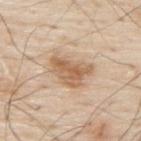imaging modality — 15 mm crop, total-body photography; lesion size — ~5 mm (longest diameter); lighting — white-light illumination; patient — male, about 80 years old; body site — the upper back.